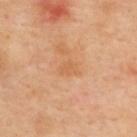workup: imaged on a skin check; not biopsied
lesion size: ~2.5 mm (longest diameter)
anatomic site: the upper back
automated lesion analysis: a footprint of about 3.5 mm², a shape eccentricity near 0.7, and a shape-asymmetry score of about 0.35 (0 = symmetric); a border-irregularity index near 3/10, a color-variation rating of about 0.5/10, and radial color variation of about 0.5; an automated nevus-likeness rating near 0 out of 100 and a detector confidence of about 100 out of 100 that the crop contains a lesion
subject: female, aged 38–42
image: 15 mm crop, total-body photography
tile lighting: cross-polarized